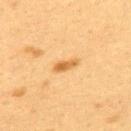Clinical impression:
Imaged during a routine full-body skin examination; the lesion was not biopsied and no histopathology is available.
Context:
Imaged with cross-polarized lighting. The lesion's longest dimension is about 3 mm. From the upper back. A region of skin cropped from a whole-body photographic capture, roughly 15 mm wide. The subject is a female approximately 40 years of age.